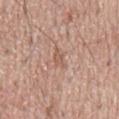body site — the mid back | lighting — white-light illumination | size — ≈3 mm | subject — male, in their mid- to late 60s | TBP lesion metrics — an area of roughly 3 mm², an eccentricity of roughly 0.85, and two-axis asymmetry of about 0.45; an average lesion color of about L≈57 a*≈20 b*≈28 (CIELAB) and roughly 7 lightness units darker than nearby skin; a color-variation rating of about 1/10 and peripheral color asymmetry of about 0.5 | image source — ~15 mm tile from a whole-body skin photo.